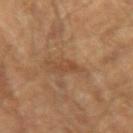biopsy status = no biopsy performed (imaged during a skin exam) | diameter = ~2.5 mm (longest diameter) | anatomic site = the left upper arm | patient = male, approximately 65 years of age | acquisition = total-body-photography crop, ~15 mm field of view.